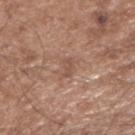Imaged during a routine full-body skin examination; the lesion was not biopsied and no histopathology is available.
A 15 mm close-up extracted from a 3D total-body photography capture.
On the right upper arm.
The tile uses white-light illumination.
Automated image analysis of the tile measured an area of roughly 2.5 mm², an outline eccentricity of about 0.85 (0 = round, 1 = elongated), and a shape-asymmetry score of about 0.4 (0 = symmetric). The analysis additionally found an average lesion color of about L≈51 a*≈19 b*≈28 (CIELAB), about 7 CIELAB-L* units darker than the surrounding skin, and a lesion-to-skin contrast of about 5 (normalized; higher = more distinct).
A male subject in their mid-70s.